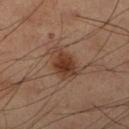workup: catalogued during a skin exam; not biopsied | patient: male, in their 60s | illumination: cross-polarized | acquisition: 15 mm crop, total-body photography | location: the right lower leg.